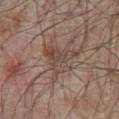{"biopsy_status": "not biopsied; imaged during a skin examination", "automated_metrics": {"area_mm2_approx": 12.0, "shape_asymmetry": 0.5, "vs_skin_darker_L": 8.0, "vs_skin_contrast_norm": 6.0, "nevus_likeness_0_100": 0, "lesion_detection_confidence_0_100": 75}, "site": "chest", "lighting": "white-light", "patient": {"sex": "male", "age_approx": 80}, "image": {"source": "total-body photography crop", "field_of_view_mm": 15}}